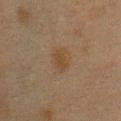Clinical impression:
The lesion was photographed on a routine skin check and not biopsied; there is no pathology result.
Context:
The lesion's longest dimension is about 3 mm. On the chest. A 15 mm crop from a total-body photograph taken for skin-cancer surveillance. This is a cross-polarized tile. A female subject, aged 38–42.